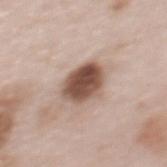The lesion was tiled from a total-body skin photograph and was not biopsied. A female patient, in their mid-60s. Imaged with white-light lighting. Measured at roughly 4.5 mm in maximum diameter. An algorithmic analysis of the crop reported a shape eccentricity near 0.6 and two-axis asymmetry of about 0.15. The software also gave about 18 CIELAB-L* units darker than the surrounding skin and a lesion-to-skin contrast of about 12 (normalized; higher = more distinct). It also reported a nevus-likeness score of about 60/100. A region of skin cropped from a whole-body photographic capture, roughly 15 mm wide. Located on the upper back.